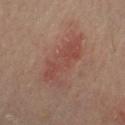<tbp_lesion>
  <biopsy_status>not biopsied; imaged during a skin examination</biopsy_status>
  <lighting>cross-polarized</lighting>
  <image>
    <source>total-body photography crop</source>
    <field_of_view_mm>15</field_of_view_mm>
  </image>
  <site>left upper arm</site>
  <patient>
    <sex>male</sex>
    <age_approx>60</age_approx>
  </patient>
  <lesion_size>
    <long_diameter_mm_approx>7.0</long_diameter_mm_approx>
  </lesion_size>
</tbp_lesion>